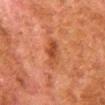Captured during whole-body skin photography for melanoma surveillance; the lesion was not biopsied.
Captured under cross-polarized illumination.
A 15 mm crop from a total-body photograph taken for skin-cancer surveillance.
A male patient roughly 80 years of age.
The lesion is on the right lower leg.
About 4 mm across.